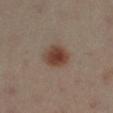No biopsy was performed on this lesion — it was imaged during a full skin examination and was not determined to be concerning. A 15 mm crop from a total-body photograph taken for skin-cancer surveillance. The lesion is on the leg. This is a cross-polarized tile. Measured at roughly 3.5 mm in maximum diameter. The subject is a female aged 38 to 42.